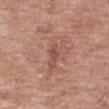Measured at roughly 2.5 mm in maximum diameter.
From the right upper arm.
The lesion-visualizer software estimated a lesion area of about 2 mm², an eccentricity of roughly 0.95, and a symmetry-axis asymmetry near 0.4. The software also gave a lesion color around L≈49 a*≈24 b*≈25 in CIELAB, about 8 CIELAB-L* units darker than the surrounding skin, and a lesion-to-skin contrast of about 6.5 (normalized; higher = more distinct). The analysis additionally found a nevus-likeness score of about 0/100 and lesion-presence confidence of about 70/100.
The patient is a female roughly 70 years of age.
This image is a 15 mm lesion crop taken from a total-body photograph.